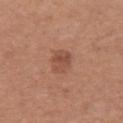No biopsy was performed on this lesion — it was imaged during a full skin examination and was not determined to be concerning. Captured under white-light illumination. The patient is a female aged 43 to 47. The lesion is located on the left upper arm. Approximately 3 mm at its widest. A region of skin cropped from a whole-body photographic capture, roughly 15 mm wide.